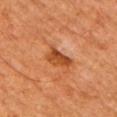Assessment:
Recorded during total-body skin imaging; not selected for excision or biopsy.
Acquisition and patient details:
The lesion is on the right upper arm. A male subject aged around 65. Cropped from a total-body skin-imaging series; the visible field is about 15 mm. Approximately 3.5 mm at its widest.